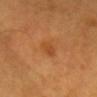The lesion was photographed on a routine skin check and not biopsied; there is no pathology result. The lesion is on the head or neck. The tile uses cross-polarized illumination. A male patient aged 58–62. A close-up tile cropped from a whole-body skin photograph, about 15 mm across. The lesion-visualizer software estimated a lesion area of about 4.5 mm², an eccentricity of roughly 0.8, and a symmetry-axis asymmetry near 0.25. The software also gave a lesion color around L≈48 a*≈26 b*≈42 in CIELAB and about 7 CIELAB-L* units darker than the surrounding skin.